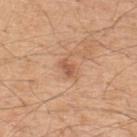{"biopsy_status": "not biopsied; imaged during a skin examination", "lighting": "white-light", "patient": {"sex": "male", "age_approx": 50}, "site": "upper back", "image": {"source": "total-body photography crop", "field_of_view_mm": 15}}